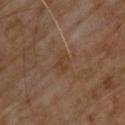Impression: No biopsy was performed on this lesion — it was imaged during a full skin examination and was not determined to be concerning. Acquisition and patient details: Located on the upper back. The subject is a male approximately 70 years of age. Imaged with cross-polarized lighting. The lesion-visualizer software estimated a footprint of about 4 mm² and two-axis asymmetry of about 0.25. It also reported an average lesion color of about L≈38 a*≈17 b*≈28 (CIELAB), roughly 5 lightness units darker than nearby skin, and a lesion-to-skin contrast of about 5.5 (normalized; higher = more distinct). The analysis additionally found a border-irregularity rating of about 2.5/10, internal color variation of about 2.5 on a 0–10 scale, and radial color variation of about 1. It also reported a classifier nevus-likeness of about 0/100 and lesion-presence confidence of about 100/100. A 15 mm crop from a total-body photograph taken for skin-cancer surveillance.